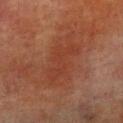Q: Was a biopsy performed?
A: total-body-photography surveillance lesion; no biopsy
Q: What kind of image is this?
A: total-body-photography crop, ~15 mm field of view
Q: Patient demographics?
A: male, in their 70s
Q: What is the lesion's diameter?
A: about 7.5 mm
Q: What did automated image analysis measure?
A: a footprint of about 16 mm² and a shape eccentricity near 0.9; an automated nevus-likeness rating near 0 out of 100
Q: Lesion location?
A: the right lower leg
Q: What lighting was used for the tile?
A: cross-polarized